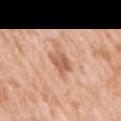Clinical impression: This lesion was catalogued during total-body skin photography and was not selected for biopsy. Clinical summary: Approximately 5 mm at its widest. From the right upper arm. A female patient, aged approximately 40. A roughly 15 mm field-of-view crop from a total-body skin photograph.